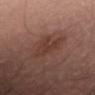Recorded during total-body skin imaging; not selected for excision or biopsy. A female patient aged around 60. On the lower back. Cropped from a total-body skin-imaging series; the visible field is about 15 mm.